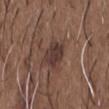{
  "biopsy_status": "not biopsied; imaged during a skin examination",
  "automated_metrics": {
    "cielab_L": 36,
    "cielab_a": 16,
    "cielab_b": 20,
    "vs_skin_darker_L": 9.0,
    "vs_skin_contrast_norm": 9.0,
    "peripheral_color_asymmetry": 1.0,
    "nevus_likeness_0_100": 25
  },
  "lesion_size": {
    "long_diameter_mm_approx": 3.0
  },
  "image": {
    "source": "total-body photography crop",
    "field_of_view_mm": 15
  },
  "site": "chest",
  "patient": {
    "sex": "male",
    "age_approx": 50
  },
  "lighting": "white-light"
}